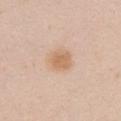Clinical impression: The lesion was photographed on a routine skin check and not biopsied; there is no pathology result. Acquisition and patient details: The lesion is located on the left upper arm. Cropped from a total-body skin-imaging series; the visible field is about 15 mm. A female subject roughly 20 years of age.